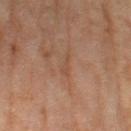Notes:
• notes — total-body-photography surveillance lesion; no biopsy
• imaging modality — total-body-photography crop, ~15 mm field of view
• anatomic site — the left thigh
• subject — female, aged approximately 60